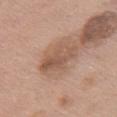No biopsy was performed on this lesion — it was imaged during a full skin examination and was not determined to be concerning.
The patient is a female aged 58 to 62.
On the chest.
Imaged with white-light lighting.
Longest diameter approximately 4.5 mm.
A close-up tile cropped from a whole-body skin photograph, about 15 mm across.